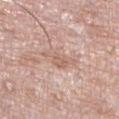A roughly 15 mm field-of-view crop from a total-body skin photograph.
Imaged with white-light lighting.
Approximately 4 mm at its widest.
The subject is a male aged approximately 75.
On the leg.